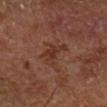Part of a total-body skin-imaging series; this lesion was reviewed on a skin check and was not flagged for biopsy.
Cropped from a total-body skin-imaging series; the visible field is about 15 mm.
Imaged with cross-polarized lighting.
The lesion is on the right lower leg.
The subject is in their mid-60s.
The total-body-photography lesion software estimated a lesion area of about 5.5 mm² and an eccentricity of roughly 0.8. The software also gave a mean CIELAB color near L≈33 a*≈22 b*≈26 and a normalized border contrast of about 6.5. It also reported an automated nevus-likeness rating near 0 out of 100.
The lesion's longest dimension is about 3.5 mm.